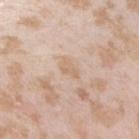Notes:
- workup — imaged on a skin check; not biopsied
- lesion diameter — ≈2.5 mm
- illumination — white-light illumination
- image source — total-body-photography crop, ~15 mm field of view
- patient — female, about 25 years old
- TBP lesion metrics — an area of roughly 3 mm², a shape eccentricity near 0.85, and a shape-asymmetry score of about 0.35 (0 = symmetric)
- location — the right upper arm